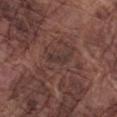Image and clinical context:
A 15 mm close-up tile from a total-body photography series done for melanoma screening. A male patient in their mid-70s. Located on the left forearm. Approximately 2.5 mm at its widest. An algorithmic analysis of the crop reported an outline eccentricity of about 0.95 (0 = round, 1 = elongated) and a shape-asymmetry score of about 0.3 (0 = symmetric). It also reported a lesion–skin lightness drop of about 5. The software also gave a nevus-likeness score of about 0/100 and a detector confidence of about 70 out of 100 that the crop contains a lesion.